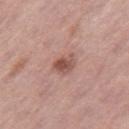Acquisition and patient details: This image is a 15 mm lesion crop taken from a total-body photograph. Located on the right thigh. The subject is a female in their 60s.